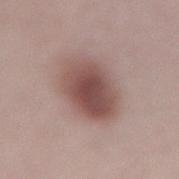Q: Is there a histopathology result?
A: catalogued during a skin exam; not biopsied
Q: What are the patient's age and sex?
A: female, aged 48–52
Q: What is the lesion's diameter?
A: about 6.5 mm
Q: What is the anatomic site?
A: the lower back
Q: What did automated image analysis measure?
A: a footprint of about 20 mm², an outline eccentricity of about 0.8 (0 = round, 1 = elongated), and a symmetry-axis asymmetry near 0.15; roughly 14 lightness units darker than nearby skin and a normalized border contrast of about 9.5; a border-irregularity index near 1.5/10, a within-lesion color-variation index near 5.5/10, and radial color variation of about 1.5; a nevus-likeness score of about 100/100 and lesion-presence confidence of about 100/100
Q: What kind of image is this?
A: 15 mm crop, total-body photography